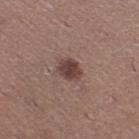Imaged during a routine full-body skin examination; the lesion was not biopsied and no histopathology is available. A 15 mm close-up tile from a total-body photography series done for melanoma screening. On the leg. The total-body-photography lesion software estimated an area of roughly 5.5 mm² and an outline eccentricity of about 0.55 (0 = round, 1 = elongated). Imaged with white-light lighting. The recorded lesion diameter is about 3 mm. A female subject, roughly 25 years of age.